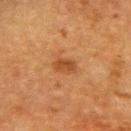Notes:
• workup · no biopsy performed (imaged during a skin exam)
• location · the upper back
• patient · female, in their mid- to late 50s
• size · ~3 mm (longest diameter)
• image source · ~15 mm crop, total-body skin-cancer survey